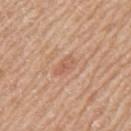Clinical impression:
Imaged during a routine full-body skin examination; the lesion was not biopsied and no histopathology is available.
Context:
Approximately 3 mm at its widest. Captured under white-light illumination. Automated tile analysis of the lesion measured an area of roughly 3.5 mm² and a symmetry-axis asymmetry near 0.25. The software also gave a lesion color around L≈59 a*≈23 b*≈32 in CIELAB, about 7 CIELAB-L* units darker than the surrounding skin, and a normalized lesion–skin contrast near 5. And it measured a border-irregularity index near 2.5/10, internal color variation of about 2.5 on a 0–10 scale, and peripheral color asymmetry of about 0.5. The software also gave a classifier nevus-likeness of about 0/100 and a detector confidence of about 100 out of 100 that the crop contains a lesion. The subject is a male roughly 80 years of age. The lesion is located on the arm. Cropped from a total-body skin-imaging series; the visible field is about 15 mm.